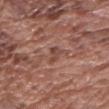Clinical impression:
The lesion was tiled from a total-body skin photograph and was not biopsied.
Image and clinical context:
On the left upper arm. A male subject, aged 73 to 77. Cropped from a whole-body photographic skin survey; the tile spans about 15 mm.